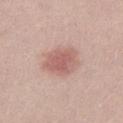Part of a total-body skin-imaging series; this lesion was reviewed on a skin check and was not flagged for biopsy. A male subject, aged 28 to 32. A 15 mm close-up tile from a total-body photography series done for melanoma screening. Measured at roughly 4 mm in maximum diameter.